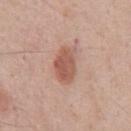<tbp_lesion>
  <biopsy_status>not biopsied; imaged during a skin examination</biopsy_status>
  <image>
    <source>total-body photography crop</source>
    <field_of_view_mm>15</field_of_view_mm>
  </image>
  <patient>
    <sex>male</sex>
    <age_approx>70</age_approx>
  </patient>
  <site>chest</site>
  <lesion_size>
    <long_diameter_mm_approx>4.5</long_diameter_mm_approx>
  </lesion_size>
  <automated_metrics>
    <border_irregularity_0_10>2.5</border_irregularity_0_10>
    <color_variation_0_10>3.0</color_variation_0_10>
    <peripheral_color_asymmetry>1.0</peripheral_color_asymmetry>
  </automated_metrics>
  <lighting>white-light</lighting>
</tbp_lesion>